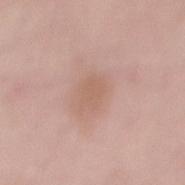Clinical impression: Recorded during total-body skin imaging; not selected for excision or biopsy. Acquisition and patient details: A roughly 15 mm field-of-view crop from a total-body skin photograph. A male subject, aged 53 to 57. The total-body-photography lesion software estimated a lesion area of about 5 mm², an eccentricity of roughly 0.75, and two-axis asymmetry of about 0.2. The software also gave a lesion-to-skin contrast of about 5 (normalized; higher = more distinct). It also reported a classifier nevus-likeness of about 5/100. From the lower back. This is a white-light tile.